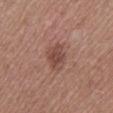This lesion was catalogued during total-body skin photography and was not selected for biopsy. This image is a 15 mm lesion crop taken from a total-body photograph. Measured at roughly 3.5 mm in maximum diameter. Located on the upper back. A female patient, in their 60s. Captured under white-light illumination.